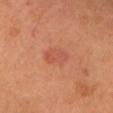| field | value |
|---|---|
| notes | no biopsy performed (imaged during a skin exam) |
| location | the head or neck |
| diameter | ~3 mm (longest diameter) |
| lighting | cross-polarized illumination |
| patient | female, aged around 65 |
| image | 15 mm crop, total-body photography |